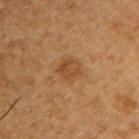Recorded during total-body skin imaging; not selected for excision or biopsy. About 3 mm across. The subject is a male aged 48 to 52. A lesion tile, about 15 mm wide, cut from a 3D total-body photograph. From the right upper arm.